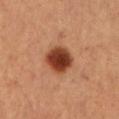{"biopsy_status": "not biopsied; imaged during a skin examination", "image": {"source": "total-body photography crop", "field_of_view_mm": 15}, "lesion_size": {"long_diameter_mm_approx": 4.0}, "patient": {"sex": "female", "age_approx": 45}, "lighting": "cross-polarized", "site": "leg", "automated_metrics": {"shape_asymmetry": 0.15, "cielab_L": 40, "cielab_a": 28, "cielab_b": 34, "vs_skin_darker_L": 18.0, "vs_skin_contrast_norm": 13.0}}